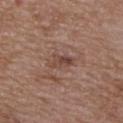Recorded during total-body skin imaging; not selected for excision or biopsy. From the back. The recorded lesion diameter is about 3.5 mm. A region of skin cropped from a whole-body photographic capture, roughly 15 mm wide. Imaged with white-light lighting. A female subject, about 65 years old.